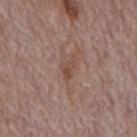Case summary:
• biopsy status — no biopsy performed (imaged during a skin exam)
• subject — male, aged around 70
• site — the mid back
• imaging modality — ~15 mm crop, total-body skin-cancer survey